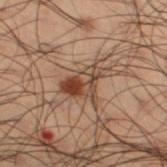No biopsy was performed on this lesion — it was imaged during a full skin examination and was not determined to be concerning.
A close-up tile cropped from a whole-body skin photograph, about 15 mm across.
A male patient, aged 48–52.
Automated tile analysis of the lesion measured an area of roughly 9.5 mm² and a shape-asymmetry score of about 0.45 (0 = symmetric).
Measured at roughly 4.5 mm in maximum diameter.
Located on the right thigh.